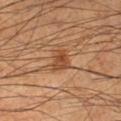Findings:
- lighting — cross-polarized illumination
- acquisition — ~15 mm crop, total-body skin-cancer survey
- subject — male, approximately 55 years of age
- lesion diameter — ≈2.5 mm
- anatomic site — the leg